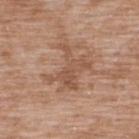Imaged during a routine full-body skin examination; the lesion was not biopsied and no histopathology is available.
The lesion-visualizer software estimated a classifier nevus-likeness of about 10/100 and a lesion-detection confidence of about 100/100.
Imaged with white-light lighting.
The lesion is located on the upper back.
The subject is a male aged around 50.
Approximately 5 mm at its widest.
A roughly 15 mm field-of-view crop from a total-body skin photograph.